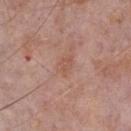{
  "biopsy_status": "not biopsied; imaged during a skin examination",
  "site": "chest",
  "image": {
    "source": "total-body photography crop",
    "field_of_view_mm": 15
  },
  "patient": {
    "sex": "male",
    "age_approx": 75
  }
}